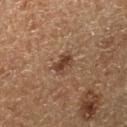{
  "biopsy_status": "not biopsied; imaged during a skin examination",
  "lesion_size": {
    "long_diameter_mm_approx": 2.5
  },
  "site": "right lower leg",
  "patient": {
    "sex": "male",
    "age_approx": 75
  },
  "image": {
    "source": "total-body photography crop",
    "field_of_view_mm": 15
  }
}